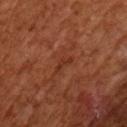acquisition: total-body-photography crop, ~15 mm field of view
patient: male, about 65 years old